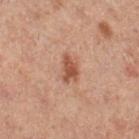The lesion was photographed on a routine skin check and not biopsied; there is no pathology result. The lesion is on the right lower leg. Measured at roughly 3 mm in maximum diameter. The subject is a male in their 60s. Imaged with cross-polarized lighting. A 15 mm close-up extracted from a 3D total-body photography capture.